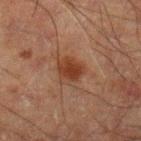On the left leg. A region of skin cropped from a whole-body photographic capture, roughly 15 mm wide. A male subject, about 60 years old.